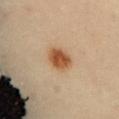follow-up: imaged on a skin check; not biopsied
tile lighting: cross-polarized illumination
acquisition: ~15 mm crop, total-body skin-cancer survey
body site: the abdomen
automated metrics: an outline eccentricity of about 0.7 (0 = round, 1 = elongated) and a symmetry-axis asymmetry near 0.15; a lesion color around L≈56 a*≈22 b*≈38 in CIELAB and roughly 13 lightness units darker than nearby skin; lesion-presence confidence of about 100/100
patient: female, about 60 years old